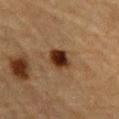No biopsy was performed on this lesion — it was imaged during a full skin examination and was not determined to be concerning.